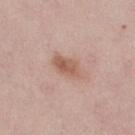Q: Is there a histopathology result?
A: total-body-photography surveillance lesion; no biopsy
Q: Automated lesion metrics?
A: a footprint of about 7 mm², a shape eccentricity near 0.8, and a shape-asymmetry score of about 0.2 (0 = symmetric)
Q: What lighting was used for the tile?
A: white-light
Q: What are the patient's age and sex?
A: female, aged 28 to 32
Q: Where on the body is the lesion?
A: the right thigh
Q: What kind of image is this?
A: ~15 mm crop, total-body skin-cancer survey
Q: How large is the lesion?
A: about 4 mm